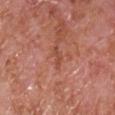| feature | finding |
|---|---|
| patient | male, approximately 65 years of age |
| anatomic site | the front of the torso |
| imaging modality | 15 mm crop, total-body photography |
| tile lighting | white-light illumination |